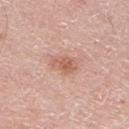  biopsy_status: not biopsied; imaged during a skin examination
  lesion_size:
    long_diameter_mm_approx: 3.0
  site: leg
  lighting: white-light
  image:
    source: total-body photography crop
    field_of_view_mm: 15
  patient:
    sex: male
    age_approx: 70